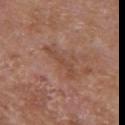The lesion was tiled from a total-body skin photograph and was not biopsied. This image is a 15 mm lesion crop taken from a total-body photograph. The lesion-visualizer software estimated a lesion area of about 4.5 mm², an eccentricity of roughly 0.95, and two-axis asymmetry of about 0.5. And it measured a lesion color around L≈47 a*≈22 b*≈28 in CIELAB, about 7 CIELAB-L* units darker than the surrounding skin, and a lesion-to-skin contrast of about 5.5 (normalized; higher = more distinct). The analysis additionally found a border-irregularity rating of about 7.5/10, internal color variation of about 0 on a 0–10 scale, and a peripheral color-asymmetry measure near 0. The tile uses white-light illumination. From the right upper arm. Measured at roughly 5 mm in maximum diameter. A male subject approximately 65 years of age.